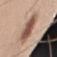<case>
<patient>
  <sex>male</sex>
  <age_approx>45</age_approx>
</patient>
<image>
  <source>total-body photography crop</source>
  <field_of_view_mm>15</field_of_view_mm>
</image>
<site>right upper arm</site>
<lesion_size>
  <long_diameter_mm_approx>7.5</long_diameter_mm_approx>
</lesion_size>
</case>